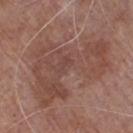A lesion tile, about 15 mm wide, cut from a 3D total-body photograph. The total-body-photography lesion software estimated a mean CIELAB color near L≈46 a*≈20 b*≈24 and a normalized lesion–skin contrast near 5.5. The software also gave a border-irregularity index near 8/10, a within-lesion color-variation index near 3.5/10, and radial color variation of about 1. The analysis additionally found an automated nevus-likeness rating near 0 out of 100 and a detector confidence of about 100 out of 100 that the crop contains a lesion. The lesion is on the chest. The tile uses white-light illumination. A male patient about 80 years old.